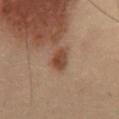Clinical impression: This lesion was catalogued during total-body skin photography and was not selected for biopsy. Background: A male patient aged 53 to 57. From the abdomen. A 15 mm close-up extracted from a 3D total-body photography capture.